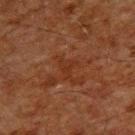biopsy status: catalogued during a skin exam; not biopsied | diameter: about 2.5 mm | image source: ~15 mm tile from a whole-body skin photo | tile lighting: cross-polarized | location: the upper back | automated metrics: a lesion area of about 3.5 mm² and a symmetry-axis asymmetry near 0.55; an automated nevus-likeness rating near 0 out of 100 and a detector confidence of about 90 out of 100 that the crop contains a lesion | patient: male, about 60 years old.